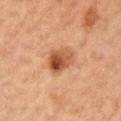Recorded during total-body skin imaging; not selected for excision or biopsy.
A 15 mm crop from a total-body photograph taken for skin-cancer surveillance.
A female subject, roughly 40 years of age.
The lesion-visualizer software estimated roughly 11 lightness units darker than nearby skin and a lesion-to-skin contrast of about 9 (normalized; higher = more distinct). The analysis additionally found border irregularity of about 2.5 on a 0–10 scale and a within-lesion color-variation index near 9/10. The analysis additionally found a nevus-likeness score of about 95/100 and a lesion-detection confidence of about 100/100.
The recorded lesion diameter is about 4.5 mm.
Captured under cross-polarized illumination.
From the left upper arm.